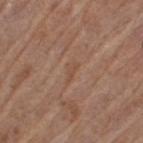Assessment: This lesion was catalogued during total-body skin photography and was not selected for biopsy. Image and clinical context: On the left thigh. This image is a 15 mm lesion crop taken from a total-body photograph. The subject is a female aged 53 to 57. Automated tile analysis of the lesion measured a footprint of about 2.5 mm², an eccentricity of roughly 0.9, and two-axis asymmetry of about 0.4. The software also gave a lesion-detection confidence of about 75/100.